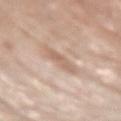<case>
  <biopsy_status>not biopsied; imaged during a skin examination</biopsy_status>
  <lighting>white-light</lighting>
  <image>
    <source>total-body photography crop</source>
    <field_of_view_mm>15</field_of_view_mm>
  </image>
  <patient>
    <sex>male</sex>
    <age_approx>80</age_approx>
  </patient>
  <site>right upper arm</site>
  <automated_metrics>
    <area_mm2_approx>5.5</area_mm2_approx>
    <shape_asymmetry>0.25</shape_asymmetry>
    <cielab_L>63</cielab_L>
    <cielab_a>17</cielab_a>
    <cielab_b>28</cielab_b>
    <vs_skin_darker_L>9.0</vs_skin_darker_L>
    <vs_skin_contrast_norm>5.5</vs_skin_contrast_norm>
    <peripheral_color_asymmetry>1.0</peripheral_color_asymmetry>
    <nevus_likeness_0_100>0</nevus_likeness_0_100>
  </automated_metrics>
</case>